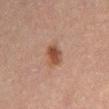Part of a total-body skin-imaging series; this lesion was reviewed on a skin check and was not flagged for biopsy. Cropped from a total-body skin-imaging series; the visible field is about 15 mm. A male subject, about 60 years old. Located on the abdomen.